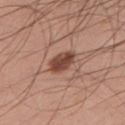Q: Who is the patient?
A: male, about 30 years old
Q: Lesion location?
A: the front of the torso
Q: How large is the lesion?
A: ~3.5 mm (longest diameter)
Q: Automated lesion metrics?
A: a footprint of about 6.5 mm²
Q: What kind of image is this?
A: total-body-photography crop, ~15 mm field of view
Q: What lighting was used for the tile?
A: white-light illumination